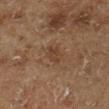Recorded during total-body skin imaging; not selected for excision or biopsy.
A male subject in their mid- to late 70s.
From the right lower leg.
A lesion tile, about 15 mm wide, cut from a 3D total-body photograph.
An algorithmic analysis of the crop reported a border-irregularity rating of about 3/10 and a within-lesion color-variation index near 2/10.
Approximately 3 mm at its widest.
This is a cross-polarized tile.